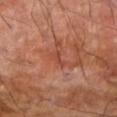biopsy status = total-body-photography surveillance lesion; no biopsy
image = ~15 mm crop, total-body skin-cancer survey
lesion diameter = ~2.5 mm (longest diameter)
subject = male, aged 68–72
anatomic site = the right forearm